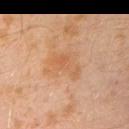Impression: This lesion was catalogued during total-body skin photography and was not selected for biopsy. Clinical summary: This image is a 15 mm lesion crop taken from a total-body photograph. On the left upper arm. A male subject aged 28 to 32.